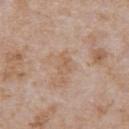No biopsy was performed on this lesion — it was imaged during a full skin examination and was not determined to be concerning. About 2.5 mm across. On the chest. Cropped from a whole-body photographic skin survey; the tile spans about 15 mm. The tile uses white-light illumination. A male subject, aged around 65.